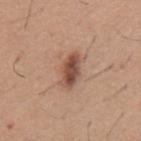lesion_size:
  long_diameter_mm_approx: 4.0
lighting: white-light
image:
  source: total-body photography crop
  field_of_view_mm: 15
site: mid back
patient:
  sex: male
  age_approx: 65